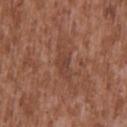Findings:
• notes — total-body-photography surveillance lesion; no biopsy
• lesion size — about 2.5 mm
• anatomic site — the upper back
• patient — male, in their mid- to late 40s
• TBP lesion metrics — a lesion area of about 3 mm², a shape eccentricity near 0.8, and two-axis asymmetry of about 0.25; a mean CIELAB color near L≈41 a*≈22 b*≈27, roughly 6 lightness units darker than nearby skin, and a lesion-to-skin contrast of about 5 (normalized; higher = more distinct); a nevus-likeness score of about 0/100 and lesion-presence confidence of about 70/100
• lighting — white-light
• image source — 15 mm crop, total-body photography